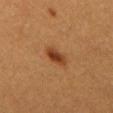The lesion is on the abdomen. Automated tile analysis of the lesion measured an area of roughly 5 mm², a shape eccentricity near 0.85, and a symmetry-axis asymmetry near 0.2. And it measured an average lesion color of about L≈38 a*≈24 b*≈34 (CIELAB), a lesion–skin lightness drop of about 11, and a normalized lesion–skin contrast near 9. A 15 mm close-up tile from a total-body photography series done for melanoma screening. A male patient aged 58–62.